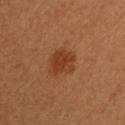Notes:
– biopsy status: no biopsy performed (imaged during a skin exam)
– diameter: ≈3 mm
– subject: female, aged 48 to 52
– illumination: cross-polarized illumination
– image source: ~15 mm tile from a whole-body skin photo